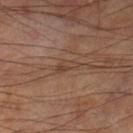{
  "biopsy_status": "not biopsied; imaged during a skin examination",
  "site": "left lower leg",
  "image": {
    "source": "total-body photography crop",
    "field_of_view_mm": 15
  },
  "automated_metrics": {
    "cielab_L": 40,
    "cielab_a": 17,
    "cielab_b": 27,
    "vs_skin_contrast_norm": 5.5
  },
  "patient": {
    "sex": "male",
    "age_approx": 70
  },
  "lighting": "cross-polarized"
}